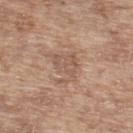<case>
<biopsy_status>not biopsied; imaged during a skin examination</biopsy_status>
<site>upper back</site>
<patient>
  <sex>male</sex>
  <age_approx>65</age_approx>
</patient>
<image>
  <source>total-body photography crop</source>
  <field_of_view_mm>15</field_of_view_mm>
</image>
</case>